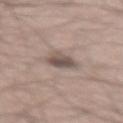notes — no biopsy performed (imaged during a skin exam) | size — about 3 mm | anatomic site — the lower back | imaging modality — ~15 mm tile from a whole-body skin photo | patient — male, in their mid-60s | TBP lesion metrics — a normalized lesion–skin contrast near 8; a border-irregularity rating of about 2.5/10 and a peripheral color-asymmetry measure near 1.5; an automated nevus-likeness rating near 35 out of 100 and a detector confidence of about 95 out of 100 that the crop contains a lesion | lighting — white-light illumination.